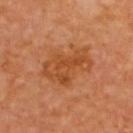• biopsy status: imaged on a skin check; not biopsied
• subject: male, about 65 years old
• imaging modality: total-body-photography crop, ~15 mm field of view
• site: the upper back
• TBP lesion metrics: an area of roughly 15 mm² and a shape eccentricity near 0.85; a mean CIELAB color near L≈48 a*≈27 b*≈40, a lesion–skin lightness drop of about 8, and a lesion-to-skin contrast of about 6.5 (normalized; higher = more distinct); internal color variation of about 4 on a 0–10 scale and radial color variation of about 1.5
• illumination: cross-polarized illumination
• lesion size: about 6 mm